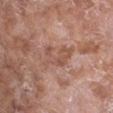The lesion was tiled from a total-body skin photograph and was not biopsied. The lesion is on the chest. A lesion tile, about 15 mm wide, cut from a 3D total-body photograph. Automated tile analysis of the lesion measured a detector confidence of about 95 out of 100 that the crop contains a lesion. The patient is a female approximately 75 years of age.